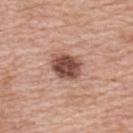The lesion was tiled from a total-body skin photograph and was not biopsied. A female subject, about 60 years old. From the back. This image is a 15 mm lesion crop taken from a total-body photograph. Automated image analysis of the tile measured an outline eccentricity of about 0.55 (0 = round, 1 = elongated) and two-axis asymmetry of about 0.15. The analysis additionally found a classifier nevus-likeness of about 75/100 and a lesion-detection confidence of about 100/100. The tile uses white-light illumination. Longest diameter approximately 3.5 mm.